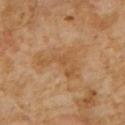{"biopsy_status": "not biopsied; imaged during a skin examination", "image": {"source": "total-body photography crop", "field_of_view_mm": 15}, "patient": {"sex": "male", "age_approx": 60}, "automated_metrics": {"area_mm2_approx": 11.0, "shape_asymmetry": 0.65, "nevus_likeness_0_100": 0, "lesion_detection_confidence_0_100": 100}, "lesion_size": {"long_diameter_mm_approx": 5.0}, "site": "chest"}